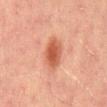Impression: The lesion was tiled from a total-body skin photograph and was not biopsied. Background: From the mid back. A region of skin cropped from a whole-body photographic capture, roughly 15 mm wide. The patient is a male about 45 years old.